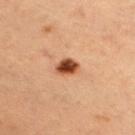follow-up: total-body-photography surveillance lesion; no biopsy | acquisition: ~15 mm crop, total-body skin-cancer survey | location: the chest | patient: female, roughly 40 years of age.